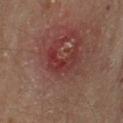Findings:
– patient: male, aged 63–67
– acquisition: total-body-photography crop, ~15 mm field of view
– body site: the left upper arm